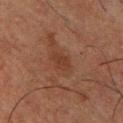Recorded during total-body skin imaging; not selected for excision or biopsy. Automated tile analysis of the lesion measured an automated nevus-likeness rating near 0 out of 100 and a detector confidence of about 100 out of 100 that the crop contains a lesion. About 2.5 mm across. From the chest. The patient is a male aged 48 to 52. Captured under cross-polarized illumination. This image is a 15 mm lesion crop taken from a total-body photograph.